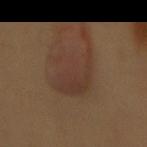notes = no biopsy performed (imaged during a skin exam)
subject = female, approximately 60 years of age
image = ~15 mm tile from a whole-body skin photo
lighting = cross-polarized
site = the mid back
size = about 6 mm